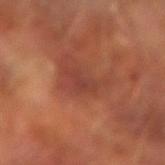biopsy status: catalogued during a skin exam; not biopsied
site: the left forearm
size: ~3 mm (longest diameter)
subject: male, approximately 65 years of age
imaging modality: 15 mm crop, total-body photography
automated lesion analysis: a shape-asymmetry score of about 0.5 (0 = symmetric); an average lesion color of about L≈38 a*≈26 b*≈27 (CIELAB), about 6 CIELAB-L* units darker than the surrounding skin, and a normalized border contrast of about 5.5; border irregularity of about 5 on a 0–10 scale, a within-lesion color-variation index near 1.5/10, and radial color variation of about 0.5; a classifier nevus-likeness of about 0/100 and a detector confidence of about 85 out of 100 that the crop contains a lesion